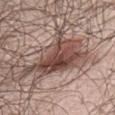This lesion was catalogued during total-body skin photography and was not selected for biopsy. The tile uses white-light illumination. A 15 mm close-up extracted from a 3D total-body photography capture. About 8 mm across. From the chest. The patient is a male aged approximately 40.